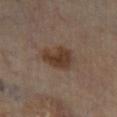biopsy status — catalogued during a skin exam; not biopsied | anatomic site — the left lower leg | illumination — cross-polarized | patient — male, in their 70s | imaging modality — ~15 mm crop, total-body skin-cancer survey.